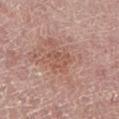biopsy_status: not biopsied; imaged during a skin examination